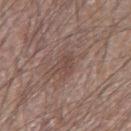Q: Was this lesion biopsied?
A: no biopsy performed (imaged during a skin exam)
Q: What is the imaging modality?
A: 15 mm crop, total-body photography
Q: What is the anatomic site?
A: the right upper arm
Q: Who is the patient?
A: male, aged 63 to 67
Q: Lesion size?
A: ≈3 mm